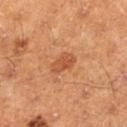The lesion was photographed on a routine skin check and not biopsied; there is no pathology result.
A male subject, in their 60s.
The lesion-visualizer software estimated an average lesion color of about L≈48 a*≈26 b*≈35 (CIELAB), a lesion–skin lightness drop of about 8, and a normalized border contrast of about 6.5. And it measured an automated nevus-likeness rating near 75 out of 100 and lesion-presence confidence of about 100/100.
A 15 mm crop from a total-body photograph taken for skin-cancer surveillance.
The lesion's longest dimension is about 3.5 mm.
Captured under cross-polarized illumination.
Located on the left lower leg.